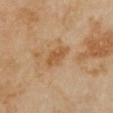{
  "biopsy_status": "not biopsied; imaged during a skin examination",
  "lesion_size": {
    "long_diameter_mm_approx": 3.5
  },
  "automated_metrics": {
    "area_mm2_approx": 5.0,
    "eccentricity": 0.85,
    "shape_asymmetry": 0.2,
    "border_irregularity_0_10": 2.0,
    "color_variation_0_10": 2.0
  },
  "site": "chest",
  "patient": {
    "sex": "female",
    "age_approx": 35
  },
  "lighting": "cross-polarized",
  "image": {
    "source": "total-body photography crop",
    "field_of_view_mm": 15
  }
}